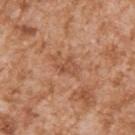  biopsy_status: not biopsied; imaged during a skin examination
  automated_metrics:
    area_mm2_approx: 4.0
    shape_asymmetry: 0.5
    color_variation_0_10: 2.0
    peripheral_color_asymmetry: 1.0
    nevus_likeness_0_100: 0
  lighting: white-light
  site: right upper arm
  lesion_size:
    long_diameter_mm_approx: 3.0
  patient:
    sex: male
    age_approx: 45
  image:
    source: total-body photography crop
    field_of_view_mm: 15